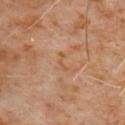notes: imaged on a skin check; not biopsied
subject: male, in their 60s
location: the chest
image source: total-body-photography crop, ~15 mm field of view